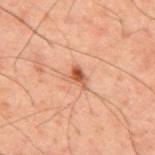Clinical impression: This lesion was catalogued during total-body skin photography and was not selected for biopsy. Clinical summary: The subject is a male aged approximately 60. Longest diameter approximately 2.5 mm. The lesion is located on the mid back. Cropped from a total-body skin-imaging series; the visible field is about 15 mm.